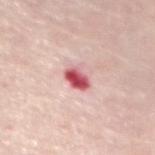Captured during whole-body skin photography for melanoma surveillance; the lesion was not biopsied. Imaged with white-light lighting. A female patient in their mid- to late 60s. A close-up tile cropped from a whole-body skin photograph, about 15 mm across. The lesion is located on the chest.